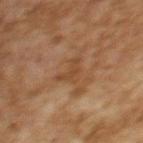image source — ~15 mm tile from a whole-body skin photo | site — the upper back | subject — female, aged around 60 | tile lighting — cross-polarized | TBP lesion metrics — an area of roughly 4.5 mm² and a shape eccentricity near 0.65; a mean CIELAB color near L≈39 a*≈18 b*≈31 and about 6 CIELAB-L* units darker than the surrounding skin; a border-irregularity rating of about 7/10 and internal color variation of about 1 on a 0–10 scale; a classifier nevus-likeness of about 0/100 and a detector confidence of about 100 out of 100 that the crop contains a lesion | lesion diameter — ≈3 mm.